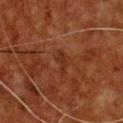Notes:
- workup: no biopsy performed (imaged during a skin exam)
- tile lighting: cross-polarized
- image source: 15 mm crop, total-body photography
- lesion diameter: about 3 mm
- patient: male, aged around 60
- body site: the chest
- image-analysis metrics: an area of roughly 3 mm² and an eccentricity of roughly 0.9; a border-irregularity rating of about 4.5/10, a color-variation rating of about 0/10, and peripheral color asymmetry of about 0